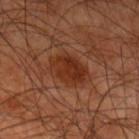Impression:
No biopsy was performed on this lesion — it was imaged during a full skin examination and was not determined to be concerning.
Clinical summary:
A close-up tile cropped from a whole-body skin photograph, about 15 mm across. The patient is a male approximately 45 years of age. From the left upper arm.